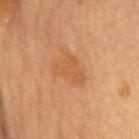Acquisition and patient details: A roughly 15 mm field-of-view crop from a total-body skin photograph. A female subject aged approximately 60. The lesion-visualizer software estimated a mean CIELAB color near L≈50 a*≈22 b*≈36 and about 6 CIELAB-L* units darker than the surrounding skin. It also reported border irregularity of about 4 on a 0–10 scale, internal color variation of about 2 on a 0–10 scale, and peripheral color asymmetry of about 1. The analysis additionally found a classifier nevus-likeness of about 0/100 and lesion-presence confidence of about 100/100. Measured at roughly 4 mm in maximum diameter. From the chest.